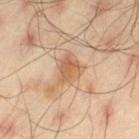Imaged during a routine full-body skin examination; the lesion was not biopsied and no histopathology is available.
The lesion is on the left thigh.
The tile uses cross-polarized illumination.
A lesion tile, about 15 mm wide, cut from a 3D total-body photograph.
The total-body-photography lesion software estimated an average lesion color of about L≈61 a*≈19 b*≈35 (CIELAB), about 9 CIELAB-L* units darker than the surrounding skin, and a normalized lesion–skin contrast near 6.5. And it measured internal color variation of about 5 on a 0–10 scale and a peripheral color-asymmetry measure near 1.5.
The subject is a male approximately 45 years of age.